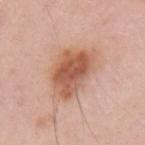Longest diameter approximately 6.5 mm. On the arm. Automated tile analysis of the lesion measured a lesion area of about 20 mm², an outline eccentricity of about 0.75 (0 = round, 1 = elongated), and a shape-asymmetry score of about 0.25 (0 = symmetric). It also reported an average lesion color of about L≈58 a*≈23 b*≈31 (CIELAB), roughly 13 lightness units darker than nearby skin, and a normalized lesion–skin contrast near 8.5. It also reported an automated nevus-likeness rating near 95 out of 100 and lesion-presence confidence of about 100/100. A female subject, in their 40s. The tile uses white-light illumination. A 15 mm close-up tile from a total-body photography series done for melanoma screening.